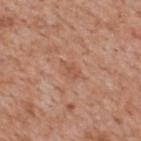The lesion was tiled from a total-body skin photograph and was not biopsied.
About 2.5 mm across.
A 15 mm close-up tile from a total-body photography series done for melanoma screening.
This is a white-light tile.
On the mid back.
An algorithmic analysis of the crop reported a footprint of about 3.5 mm², a shape eccentricity near 0.75, and a symmetry-axis asymmetry near 0.35. The software also gave a border-irregularity index near 3.5/10, a color-variation rating of about 1.5/10, and peripheral color asymmetry of about 0.5.
A male patient, roughly 65 years of age.